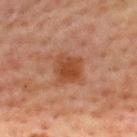Captured during whole-body skin photography for melanoma surveillance; the lesion was not biopsied.
The total-body-photography lesion software estimated an area of roughly 8 mm² and a shape eccentricity near 0.35. It also reported a lesion color around L≈38 a*≈22 b*≈30 in CIELAB, a lesion–skin lightness drop of about 8, and a lesion-to-skin contrast of about 8.5 (normalized; higher = more distinct). The software also gave a nevus-likeness score of about 70/100 and a detector confidence of about 100 out of 100 that the crop contains a lesion.
This is a cross-polarized tile.
A male patient in their 60s.
Measured at roughly 3 mm in maximum diameter.
Cropped from a whole-body photographic skin survey; the tile spans about 15 mm.
Located on the back.